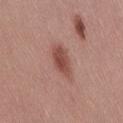{"biopsy_status": "not biopsied; imaged during a skin examination", "site": "lower back", "lighting": "white-light", "lesion_size": {"long_diameter_mm_approx": 4.5}, "image": {"source": "total-body photography crop", "field_of_view_mm": 15}, "patient": {"sex": "female", "age_approx": 25}}